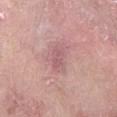Impression:
Part of a total-body skin-imaging series; this lesion was reviewed on a skin check and was not flagged for biopsy.
Context:
The lesion-visualizer software estimated border irregularity of about 4 on a 0–10 scale and a peripheral color-asymmetry measure near 0.5. The lesion's longest dimension is about 4 mm. The lesion is located on the left forearm. The patient is a female approximately 70 years of age. A close-up tile cropped from a whole-body skin photograph, about 15 mm across.